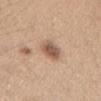<case>
<biopsy_status>not biopsied; imaged during a skin examination</biopsy_status>
<patient>
  <sex>female</sex>
  <age_approx>40</age_approx>
</patient>
<site>head or neck</site>
<lesion_size>
  <long_diameter_mm_approx>3.0</long_diameter_mm_approx>
</lesion_size>
<image>
  <source>total-body photography crop</source>
  <field_of_view_mm>15</field_of_view_mm>
</image>
</case>